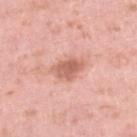Findings:
• acquisition · ~15 mm tile from a whole-body skin photo
• illumination · white-light
• image-analysis metrics · a footprint of about 7 mm² and two-axis asymmetry of about 0.25; a lesion-to-skin contrast of about 7.5 (normalized; higher = more distinct); a border-irregularity rating of about 3/10 and internal color variation of about 3 on a 0–10 scale
• location · the right lower leg
• patient · female, about 40 years old